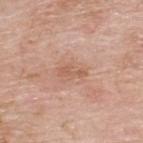biopsy status=catalogued during a skin exam; not biopsied.